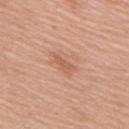Findings:
• follow-up · catalogued during a skin exam; not biopsied
• anatomic site · the upper back
• automated metrics · a border-irregularity index near 4/10 and radial color variation of about 0.5; an automated nevus-likeness rating near 0 out of 100 and lesion-presence confidence of about 100/100
• illumination · white-light illumination
• image · ~15 mm crop, total-body skin-cancer survey
• patient · female, aged 73–77